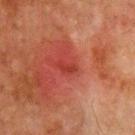<record>
  <biopsy_status>not biopsied; imaged during a skin examination</biopsy_status>
  <lesion_size>
    <long_diameter_mm_approx>2.0</long_diameter_mm_approx>
  </lesion_size>
  <lighting>cross-polarized</lighting>
  <image>
    <source>total-body photography crop</source>
    <field_of_view_mm>15</field_of_view_mm>
  </image>
  <site>front of the torso</site>
  <patient>
    <sex>male</sex>
    <age_approx>65</age_approx>
  </patient>
  <automated_metrics>
    <cielab_L>32</cielab_L>
    <cielab_a>32</cielab_a>
    <cielab_b>28</cielab_b>
    <vs_skin_contrast_norm>6.0</vs_skin_contrast_norm>
  </automated_metrics>
</record>